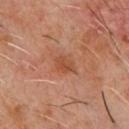biopsy_status: not biopsied; imaged during a skin examination
patient:
  sex: male
  age_approx: 60
image:
  source: total-body photography crop
  field_of_view_mm: 15
site: chest